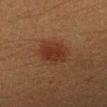Context: The patient is a male aged 38–42. On the left forearm. This image is a 15 mm lesion crop taken from a total-body photograph. The lesion-visualizer software estimated an outline eccentricity of about 0.5 (0 = round, 1 = elongated). The software also gave a mean CIELAB color near L≈26 a*≈20 b*≈25, a lesion–skin lightness drop of about 7, and a lesion-to-skin contrast of about 7.5 (normalized; higher = more distinct). Approximately 3.5 mm at its widest.